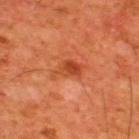Findings:
- biopsy status — total-body-photography surveillance lesion; no biopsy
- subject — male, approximately 60 years of age
- tile lighting — cross-polarized
- image — total-body-photography crop, ~15 mm field of view
- location — the upper back